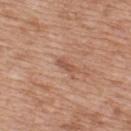Captured during whole-body skin photography for melanoma surveillance; the lesion was not biopsied. A lesion tile, about 15 mm wide, cut from a 3D total-body photograph. On the upper back. The patient is a male roughly 50 years of age. An algorithmic analysis of the crop reported a classifier nevus-likeness of about 0/100 and a lesion-detection confidence of about 100/100. Approximately 2.5 mm at its widest. This is a white-light tile.